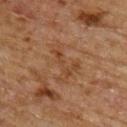Clinical impression:
The lesion was tiled from a total-body skin photograph and was not biopsied.
Image and clinical context:
A lesion tile, about 15 mm wide, cut from a 3D total-body photograph. A male subject, roughly 65 years of age. From the upper back. The tile uses cross-polarized illumination. About 5 mm across.